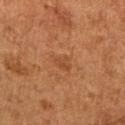  biopsy_status: not biopsied; imaged during a skin examination
  site: arm
  lighting: cross-polarized
  lesion_size:
    long_diameter_mm_approx: 3.0
  image:
    source: total-body photography crop
    field_of_view_mm: 15
  patient:
    sex: female
    age_approx: 60